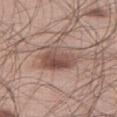Assessment: This lesion was catalogued during total-body skin photography and was not selected for biopsy. Clinical summary: The lesion's longest dimension is about 5.5 mm. A male patient approximately 60 years of age. Automated tile analysis of the lesion measured an automated nevus-likeness rating near 90 out of 100 and a detector confidence of about 100 out of 100 that the crop contains a lesion. The lesion is located on the leg. A 15 mm close-up tile from a total-body photography series done for melanoma screening. The tile uses white-light illumination.